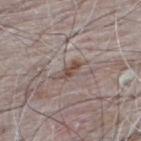No biopsy was performed on this lesion — it was imaged during a full skin examination and was not determined to be concerning. An algorithmic analysis of the crop reported an area of roughly 3 mm² and a symmetry-axis asymmetry near 0.3. The analysis additionally found a within-lesion color-variation index near 1.5/10 and radial color variation of about 0.5. Approximately 3 mm at its widest. On the upper back. Cropped from a whole-body photographic skin survey; the tile spans about 15 mm. A male subject aged 63–67.